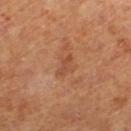| field | value |
|---|---|
| workup | total-body-photography surveillance lesion; no biopsy |
| lighting | cross-polarized |
| patient | female, aged 63–67 |
| acquisition | 15 mm crop, total-body photography |
| image-analysis metrics | a mean CIELAB color near L≈47 a*≈25 b*≈33, about 7 CIELAB-L* units darker than the surrounding skin, and a lesion-to-skin contrast of about 5.5 (normalized; higher = more distinct); border irregularity of about 5 on a 0–10 scale and a peripheral color-asymmetry measure near 0; an automated nevus-likeness rating near 0 out of 100 and lesion-presence confidence of about 100/100 |
| anatomic site | the right lower leg |